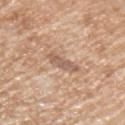A male patient about 65 years old. A region of skin cropped from a whole-body photographic capture, roughly 15 mm wide. The lesion is on the arm. The recorded lesion diameter is about 3.5 mm.